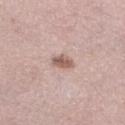{
  "biopsy_status": "not biopsied; imaged during a skin examination",
  "patient": {
    "sex": "female",
    "age_approx": 65
  },
  "site": "left lower leg",
  "lighting": "white-light",
  "image": {
    "source": "total-body photography crop",
    "field_of_view_mm": 15
  },
  "automated_metrics": {
    "cielab_L": 59,
    "cielab_a": 18,
    "cielab_b": 24,
    "vs_skin_darker_L": 12.0,
    "vs_skin_contrast_norm": 8.0,
    "border_irregularity_0_10": 1.5,
    "color_variation_0_10": 3.0,
    "peripheral_color_asymmetry": 1.0
  }
}